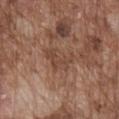Clinical summary:
Approximately 4 mm at its widest. A region of skin cropped from a whole-body photographic capture, roughly 15 mm wide. From the chest. A male subject in their mid-70s. This is a white-light tile.